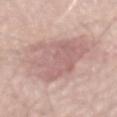lesion diameter = about 9 mm | image-analysis metrics = two-axis asymmetry of about 0.35; a border-irregularity index near 5/10, internal color variation of about 3 on a 0–10 scale, and peripheral color asymmetry of about 1; a classifier nevus-likeness of about 0/100 and a lesion-detection confidence of about 95/100 | subject = male, about 70 years old | acquisition = ~15 mm tile from a whole-body skin photo | location = the mid back.